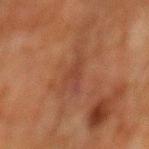- workup · total-body-photography surveillance lesion; no biopsy
- image · ~15 mm tile from a whole-body skin photo
- size · ≈4.5 mm
- patient · male, about 80 years old
- anatomic site · the mid back